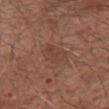The lesion was photographed on a routine skin check and not biopsied; there is no pathology result.